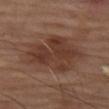Q: Is there a histopathology result?
A: no biopsy performed (imaged during a skin exam)
Q: What lighting was used for the tile?
A: cross-polarized illumination
Q: Lesion size?
A: ~7.5 mm (longest diameter)
Q: What is the imaging modality?
A: ~15 mm tile from a whole-body skin photo
Q: Where on the body is the lesion?
A: the left thigh
Q: Who is the patient?
A: male, aged around 70